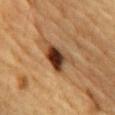{"image": {"source": "total-body photography crop", "field_of_view_mm": 15}, "lesion_size": {"long_diameter_mm_approx": 4.5}, "patient": {"sex": "male", "age_approx": "85+"}, "automated_metrics": {"eccentricity": 0.75, "shape_asymmetry": 0.4, "border_irregularity_0_10": 4.0, "peripheral_color_asymmetry": 2.5}, "site": "front of the torso", "lighting": "cross-polarized"}